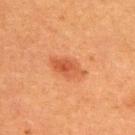The lesion was tiled from a total-body skin photograph and was not biopsied.
A male subject, in their 50s.
A close-up tile cropped from a whole-body skin photograph, about 15 mm across.
Located on the upper back.